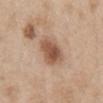– biopsy status: imaged on a skin check; not biopsied
– acquisition: ~15 mm tile from a whole-body skin photo
– lighting: white-light
– subject: female, roughly 40 years of age
– lesion diameter: about 4 mm
– site: the chest